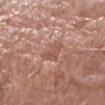biopsy status — total-body-photography surveillance lesion; no biopsy
acquisition — total-body-photography crop, ~15 mm field of view
lighting — white-light illumination
patient — male, approximately 80 years of age
automated lesion analysis — an area of roughly 5 mm², an outline eccentricity of about 0.65 (0 = round, 1 = elongated), and a shape-asymmetry score of about 0.35 (0 = symmetric); an average lesion color of about L≈54 a*≈22 b*≈28 (CIELAB), a lesion–skin lightness drop of about 7, and a lesion-to-skin contrast of about 5.5 (normalized; higher = more distinct); border irregularity of about 3.5 on a 0–10 scale and peripheral color asymmetry of about 1; an automated nevus-likeness rating near 0 out of 100 and a detector confidence of about 100 out of 100 that the crop contains a lesion
size — about 3 mm
anatomic site — the right forearm